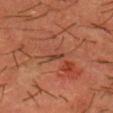notes: total-body-photography surveillance lesion; no biopsy | image-analysis metrics: an area of roughly 1.5 mm² and an outline eccentricity of about 0.95 (0 = round, 1 = elongated); a lesion color around L≈41 a*≈25 b*≈31 in CIELAB and a lesion-to-skin contrast of about 7 (normalized; higher = more distinct); a border-irregularity index near 3.5/10, internal color variation of about 0 on a 0–10 scale, and peripheral color asymmetry of about 0; a nevus-likeness score of about 0/100 | body site: the head or neck | lesion size: ~2 mm (longest diameter) | subject: male, aged approximately 40 | lighting: cross-polarized | image source: ~15 mm crop, total-body skin-cancer survey.